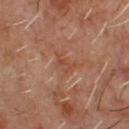Recorded during total-body skin imaging; not selected for excision or biopsy. The patient is a male aged approximately 60. From the chest. An algorithmic analysis of the crop reported a border-irregularity index near 4.5/10, internal color variation of about 2 on a 0–10 scale, and radial color variation of about 1. Imaged with cross-polarized lighting. Longest diameter approximately 3 mm. A 15 mm close-up extracted from a 3D total-body photography capture.